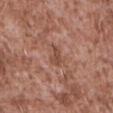biopsy status=no biopsy performed (imaged during a skin exam) | imaging modality=15 mm crop, total-body photography | body site=the front of the torso | size=about 2.5 mm | tile lighting=white-light illumination | patient=male, aged around 45.